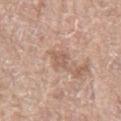Clinical summary:
The subject is a female in their 60s. The lesion is on the left thigh. Cropped from a whole-body photographic skin survey; the tile spans about 15 mm. Captured under white-light illumination.